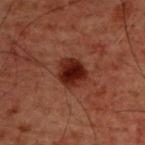The lesion was photographed on a routine skin check and not biopsied; there is no pathology result.